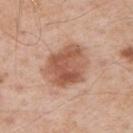patient = male, aged 53 to 57
lesion size = about 6 mm
imaging modality = ~15 mm crop, total-body skin-cancer survey
body site = the left upper arm